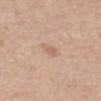<lesion>
<biopsy_status>not biopsied; imaged during a skin examination</biopsy_status>
<site>upper back</site>
<patient>
  <sex>female</sex>
  <age_approx>65</age_approx>
</patient>
<image>
  <source>total-body photography crop</source>
  <field_of_view_mm>15</field_of_view_mm>
</image>
<lighting>white-light</lighting>
</lesion>